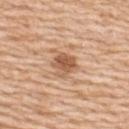A close-up tile cropped from a whole-body skin photograph, about 15 mm across.
From the back.
Longest diameter approximately 3 mm.
Imaged with white-light lighting.
The lesion-visualizer software estimated an area of roughly 6 mm², an outline eccentricity of about 0.3 (0 = round, 1 = elongated), and a shape-asymmetry score of about 0.25 (0 = symmetric). The analysis additionally found a lesion–skin lightness drop of about 12 and a normalized border contrast of about 8.
A male patient, approximately 60 years of age.